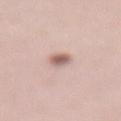Imaged during a routine full-body skin examination; the lesion was not biopsied and no histopathology is available.